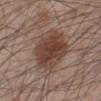<case>
<biopsy_status>not biopsied; imaged during a skin examination</biopsy_status>
<site>left lower leg</site>
<patient>
  <sex>male</sex>
  <age_approx>60</age_approx>
</patient>
<image>
  <source>total-body photography crop</source>
  <field_of_view_mm>15</field_of_view_mm>
</image>
</case>